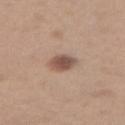Findings:
* anatomic site — the right thigh
* lighting — white-light illumination
* lesion size — ≈3 mm
* acquisition — total-body-photography crop, ~15 mm field of view
* subject — female, approximately 40 years of age